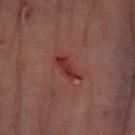This lesion was catalogued during total-body skin photography and was not selected for biopsy.
A 15 mm close-up extracted from a 3D total-body photography capture.
Automated image analysis of the tile measured an average lesion color of about L≈33 a*≈29 b*≈23 (CIELAB) and a lesion–skin lightness drop of about 8. The analysis additionally found a nevus-likeness score of about 0/100 and lesion-presence confidence of about 100/100.
A male patient approximately 65 years of age.
Located on the leg.
Imaged with cross-polarized lighting.